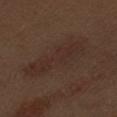follow-up = total-body-photography surveillance lesion; no biopsy | automated metrics = a border-irregularity index near 4.5/10, a color-variation rating of about 3/10, and a peripheral color-asymmetry measure near 1 | image = ~15 mm tile from a whole-body skin photo | illumination = white-light illumination | subject = male, approximately 70 years of age | size = ~9 mm (longest diameter) | site = the arm.